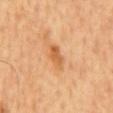| key | value |
|---|---|
| follow-up | no biopsy performed (imaged during a skin exam) |
| subject | male, in their mid-60s |
| anatomic site | the mid back |
| size | ≈3.5 mm |
| illumination | cross-polarized |
| acquisition | ~15 mm crop, total-body skin-cancer survey |